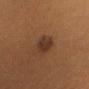{"lesion_size": {"long_diameter_mm_approx": 3.0}, "site": "head or neck", "lighting": "cross-polarized", "image": {"source": "total-body photography crop", "field_of_view_mm": 15}, "patient": {"sex": "female", "age_approx": 30}, "automated_metrics": {"area_mm2_approx": 6.0, "eccentricity": 0.6, "shape_asymmetry": 0.15, "cielab_L": 30, "cielab_a": 18, "cielab_b": 25, "vs_skin_contrast_norm": 9.0, "nevus_likeness_0_100": 95}}